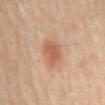Captured during whole-body skin photography for melanoma surveillance; the lesion was not biopsied.